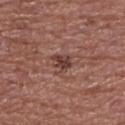notes: imaged on a skin check; not biopsied